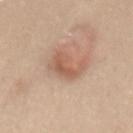Q: Is there a histopathology result?
A: imaged on a skin check; not biopsied
Q: Where on the body is the lesion?
A: the back
Q: Illumination type?
A: white-light
Q: How was this image acquired?
A: 15 mm crop, total-body photography
Q: Patient demographics?
A: female, approximately 35 years of age
Q: What did automated image analysis measure?
A: an outline eccentricity of about 0.85 (0 = round, 1 = elongated) and a shape-asymmetry score of about 0.4 (0 = symmetric); a border-irregularity index near 5.5/10 and internal color variation of about 3 on a 0–10 scale; a classifier nevus-likeness of about 65/100 and a lesion-detection confidence of about 100/100